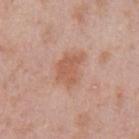Imaged during a routine full-body skin examination; the lesion was not biopsied and no histopathology is available.